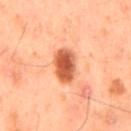This lesion was catalogued during total-body skin photography and was not selected for biopsy. Approximately 4.5 mm at its widest. The total-body-photography lesion software estimated an area of roughly 9 mm² and an outline eccentricity of about 0.85 (0 = round, 1 = elongated). It also reported a border-irregularity index near 2/10, a color-variation rating of about 4/10, and radial color variation of about 1. The tile uses cross-polarized illumination. A roughly 15 mm field-of-view crop from a total-body skin photograph. The lesion is on the mid back. A male patient, roughly 60 years of age.